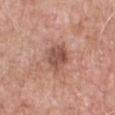follow-up=no biopsy performed (imaged during a skin exam) | lesion diameter=≈3.5 mm | lighting=white-light | subject=male, in their 60s | TBP lesion metrics=a lesion color around L≈51 a*≈23 b*≈26 in CIELAB, a lesion–skin lightness drop of about 11, and a normalized border contrast of about 8; a classifier nevus-likeness of about 0/100 and a detector confidence of about 100 out of 100 that the crop contains a lesion | image source=~15 mm tile from a whole-body skin photo | anatomic site=the front of the torso.